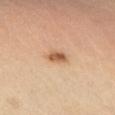Q: Illumination type?
A: cross-polarized illumination
Q: What is the lesion's diameter?
A: about 2.5 mm
Q: Where on the body is the lesion?
A: the left lower leg
Q: Automated lesion metrics?
A: a lesion color around L≈59 a*≈21 b*≈36 in CIELAB and a lesion-to-skin contrast of about 8.5 (normalized; higher = more distinct)
Q: How was this image acquired?
A: 15 mm crop, total-body photography
Q: Patient demographics?
A: male, aged 53–57